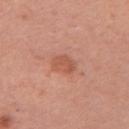Q: Was a biopsy performed?
A: total-body-photography surveillance lesion; no biopsy
Q: How was the tile lit?
A: white-light illumination
Q: Who is the patient?
A: female, roughly 35 years of age
Q: What did automated image analysis measure?
A: a nevus-likeness score of about 20/100 and a detector confidence of about 100 out of 100 that the crop contains a lesion
Q: What is the imaging modality?
A: 15 mm crop, total-body photography
Q: What is the anatomic site?
A: the left upper arm
Q: What is the lesion's diameter?
A: ≈2.5 mm